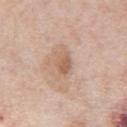workup = total-body-photography surveillance lesion; no biopsy
size = ~3 mm (longest diameter)
acquisition = ~15 mm tile from a whole-body skin photo
patient = male, aged approximately 75
anatomic site = the front of the torso
automated metrics = a lesion area of about 5 mm², an eccentricity of roughly 0.75, and a symmetry-axis asymmetry near 0.3; a classifier nevus-likeness of about 5/100 and a lesion-detection confidence of about 100/100
illumination = white-light illumination